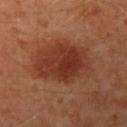Q: How large is the lesion?
A: ≈7 mm
Q: Lesion location?
A: the left upper arm
Q: Patient demographics?
A: female, aged approximately 40
Q: What kind of image is this?
A: ~15 mm crop, total-body skin-cancer survey
Q: What did the biopsy show?
A: a compound melanocytic nevus